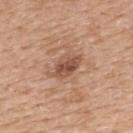Impression: This lesion was catalogued during total-body skin photography and was not selected for biopsy. Image and clinical context: On the upper back. The subject is a female approximately 55 years of age. Approximately 4.5 mm at its widest. A 15 mm close-up tile from a total-body photography series done for melanoma screening.